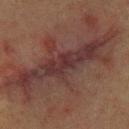This lesion was catalogued during total-body skin photography and was not selected for biopsy. This image is a 15 mm lesion crop taken from a total-body photograph. A female patient, about 40 years old. The lesion is located on the upper back.